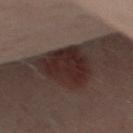Q: Was this lesion biopsied?
A: imaged on a skin check; not biopsied
Q: What did automated image analysis measure?
A: an area of roughly 23 mm², an eccentricity of roughly 0.6, and two-axis asymmetry of about 0.25; a classifier nevus-likeness of about 100/100 and lesion-presence confidence of about 80/100
Q: What is the imaging modality?
A: ~15 mm tile from a whole-body skin photo
Q: What are the patient's age and sex?
A: female, in their 50s
Q: Lesion location?
A: the right thigh